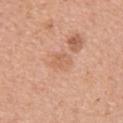Q: Patient demographics?
A: female, aged around 35
Q: Lesion location?
A: the left upper arm
Q: Illumination type?
A: white-light illumination
Q: What kind of image is this?
A: 15 mm crop, total-body photography